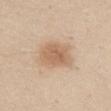Assessment: Recorded during total-body skin imaging; not selected for excision or biopsy. Context: A female subject about 40 years old. The lesion's longest dimension is about 4.5 mm. On the abdomen. An algorithmic analysis of the crop reported an outline eccentricity of about 0.75 (0 = round, 1 = elongated) and two-axis asymmetry of about 0.25. It also reported border irregularity of about 2.5 on a 0–10 scale and a color-variation rating of about 3/10. The analysis additionally found a nevus-likeness score of about 75/100 and lesion-presence confidence of about 100/100. A roughly 15 mm field-of-view crop from a total-body skin photograph.